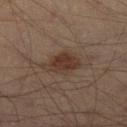Q: Was this lesion biopsied?
A: imaged on a skin check; not biopsied
Q: What is the anatomic site?
A: the right lower leg
Q: Patient demographics?
A: male, in their 40s
Q: What lighting was used for the tile?
A: cross-polarized
Q: What is the imaging modality?
A: ~15 mm crop, total-body skin-cancer survey
Q: What is the lesion's diameter?
A: ≈5 mm